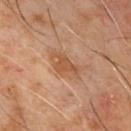biopsy status=total-body-photography surveillance lesion; no biopsy
anatomic site=the chest
lesion size=~4.5 mm (longest diameter)
subject=male, approximately 60 years of age
automated lesion analysis=a mean CIELAB color near L≈50 a*≈20 b*≈33, a lesion–skin lightness drop of about 8, and a normalized lesion–skin contrast near 6.5
tile lighting=cross-polarized illumination
acquisition=~15 mm crop, total-body skin-cancer survey